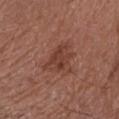subject: female, aged 78 to 82
site: the right forearm
lesion diameter: ≈4 mm
image source: 15 mm crop, total-body photography